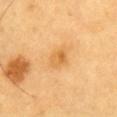notes: no biopsy performed (imaged during a skin exam).